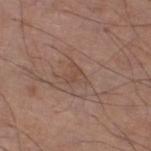| feature | finding |
|---|---|
| diameter | ≈2.5 mm |
| patient | male, in their 70s |
| image source | ~15 mm tile from a whole-body skin photo |
| image-analysis metrics | a lesion color around L≈47 a*≈18 b*≈25 in CIELAB and roughly 5 lightness units darker than nearby skin; border irregularity of about 5 on a 0–10 scale, a color-variation rating of about 2/10, and radial color variation of about 0.5; a classifier nevus-likeness of about 0/100 and a detector confidence of about 95 out of 100 that the crop contains a lesion |
| tile lighting | white-light |
| site | the left lower leg |